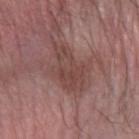The lesion was tiled from a total-body skin photograph and was not biopsied. A male patient, roughly 60 years of age. The total-body-photography lesion software estimated a lesion area of about 12 mm² and a shape eccentricity near 0.85. And it measured roughly 8 lightness units darker than nearby skin and a normalized lesion–skin contrast near 6.5. Measured at roughly 5.5 mm in maximum diameter. A close-up tile cropped from a whole-body skin photograph, about 15 mm across. The lesion is on the left forearm.